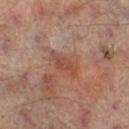Assessment: The lesion was tiled from a total-body skin photograph and was not biopsied. Image and clinical context: On the right lower leg. A 15 mm close-up extracted from a 3D total-body photography capture. The subject is a male aged 63 to 67.